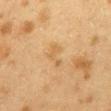Clinical summary:
Captured under cross-polarized illumination. From the mid back. The subject is a female aged around 40. This image is a 15 mm lesion crop taken from a total-body photograph.